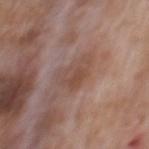{"biopsy_status": "not biopsied; imaged during a skin examination", "patient": {"sex": "male", "age_approx": 70}, "site": "back", "lighting": "white-light", "image": {"source": "total-body photography crop", "field_of_view_mm": 15}}